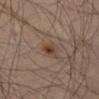No biopsy was performed on this lesion — it was imaged during a full skin examination and was not determined to be concerning. Located on the left thigh. The recorded lesion diameter is about 2 mm. A roughly 15 mm field-of-view crop from a total-body skin photograph. This is a cross-polarized tile. A male subject, aged 63 to 67.